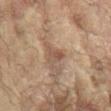Q: Was a biopsy performed?
A: imaged on a skin check; not biopsied
Q: What did automated image analysis measure?
A: a border-irregularity index near 2.5/10, internal color variation of about 3 on a 0–10 scale, and peripheral color asymmetry of about 1
Q: How large is the lesion?
A: ~2.5 mm (longest diameter)
Q: Lesion location?
A: the right arm
Q: What is the imaging modality?
A: 15 mm crop, total-body photography
Q: Illumination type?
A: cross-polarized
Q: What are the patient's age and sex?
A: female, roughly 80 years of age